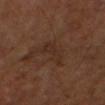workup: total-body-photography surveillance lesion; no biopsy | patient: male, roughly 65 years of age | imaging modality: ~15 mm crop, total-body skin-cancer survey | size: about 4.5 mm | location: the right forearm.